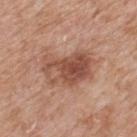Q: Who is the patient?
A: male, aged around 60
Q: Where on the body is the lesion?
A: the back
Q: Lesion size?
A: about 6 mm
Q: How was the tile lit?
A: white-light illumination
Q: How was this image acquired?
A: ~15 mm crop, total-body skin-cancer survey